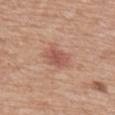Image and clinical context: Located on the mid back. A lesion tile, about 15 mm wide, cut from a 3D total-body photograph. The subject is a male aged approximately 80. Imaged with white-light lighting.